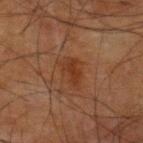Measured at roughly 4 mm in maximum diameter. A male subject, in their mid-70s. The lesion is located on the right upper arm. Cropped from a whole-body photographic skin survey; the tile spans about 15 mm.